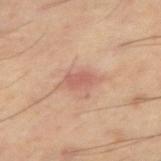About 4 mm across.
A close-up tile cropped from a whole-body skin photograph, about 15 mm across.
A male patient, about 60 years old.
The lesion is on the right thigh.